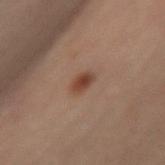biopsy status: catalogued during a skin exam; not biopsied | lighting: cross-polarized illumination | imaging modality: ~15 mm tile from a whole-body skin photo | location: the leg | TBP lesion metrics: an area of roughly 3.5 mm², a shape eccentricity near 0.85, and a symmetry-axis asymmetry near 0.25; a border-irregularity rating of about 2/10, a within-lesion color-variation index near 3.5/10, and radial color variation of about 1 | size: ≈3 mm | subject: male, aged 63 to 67.